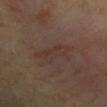<case>
<biopsy_status>not biopsied; imaged during a skin examination</biopsy_status>
<image>
  <source>total-body photography crop</source>
  <field_of_view_mm>15</field_of_view_mm>
</image>
<automated_metrics>
  <area_mm2_approx>7.5</area_mm2_approx>
  <eccentricity>0.95</eccentricity>
  <shape_asymmetry>0.3</shape_asymmetry>
  <cielab_L>33</cielab_L>
  <cielab_a>15</cielab_a>
  <cielab_b>22</cielab_b>
  <vs_skin_darker_L>4.0</vs_skin_darker_L>
  <vs_skin_contrast_norm>4.5</vs_skin_contrast_norm>
  <border_irregularity_0_10>5.0</border_irregularity_0_10>
  <color_variation_0_10>2.0</color_variation_0_10>
  <peripheral_color_asymmetry>0.5</peripheral_color_asymmetry>
</automated_metrics>
<lighting>cross-polarized</lighting>
<patient>
  <sex>male</sex>
  <age_approx>45</age_approx>
</patient>
<site>left upper arm</site>
</case>